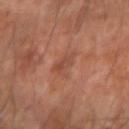  biopsy_status: not biopsied; imaged during a skin examination
  patient:
    sex: male
    age_approx: 60
  lesion_size:
    long_diameter_mm_approx: 3.0
  lighting: cross-polarized
  site: arm
  automated_metrics:
    cielab_L: 46
    cielab_a: 24
    cielab_b: 29
    vs_skin_contrast_norm: 5.0
    border_irregularity_0_10: 4.5
    peripheral_color_asymmetry: 1.0
  image:
    source: total-body photography crop
    field_of_view_mm: 15